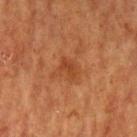Q: What is the imaging modality?
A: 15 mm crop, total-body photography
Q: Lesion location?
A: the left upper arm
Q: What are the patient's age and sex?
A: female, aged 48 to 52
Q: What did automated image analysis measure?
A: an eccentricity of roughly 0.75 and a symmetry-axis asymmetry near 0.4; an average lesion color of about L≈35 a*≈23 b*≈32 (CIELAB); border irregularity of about 4 on a 0–10 scale, a within-lesion color-variation index near 1/10, and radial color variation of about 0.5; a classifier nevus-likeness of about 0/100